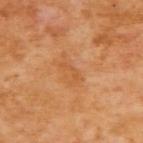Q: Was a biopsy performed?
A: total-body-photography surveillance lesion; no biopsy
Q: Illumination type?
A: cross-polarized illumination
Q: Lesion location?
A: the upper back
Q: What are the patient's age and sex?
A: female, roughly 55 years of age
Q: What is the imaging modality?
A: ~15 mm crop, total-body skin-cancer survey
Q: What did automated image analysis measure?
A: a nevus-likeness score of about 0/100 and a detector confidence of about 100 out of 100 that the crop contains a lesion
Q: What is the lesion's diameter?
A: about 2.5 mm